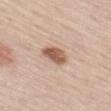Clinical impression: The lesion was photographed on a routine skin check and not biopsied; there is no pathology result. Context: A region of skin cropped from a whole-body photographic capture, roughly 15 mm wide. Approximately 3.5 mm at its widest. Imaged with white-light lighting. A male subject aged 58–62. From the left thigh.